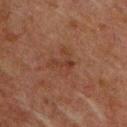Recorded during total-body skin imaging; not selected for excision or biopsy. The lesion is on the chest. A male subject aged around 60. Cropped from a whole-body photographic skin survey; the tile spans about 15 mm.